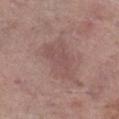workup = total-body-photography surveillance lesion; no biopsy